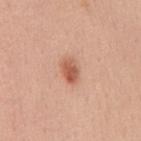Notes:
• follow-up — catalogued during a skin exam; not biopsied
• image source — ~15 mm tile from a whole-body skin photo
• patient — female, aged 38–42
• diameter — ~3 mm (longest diameter)
• lighting — white-light
• body site — the mid back